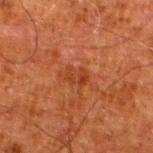Clinical impression: The lesion was photographed on a routine skin check and not biopsied; there is no pathology result. Background: Longest diameter approximately 2.5 mm. A male subject, aged around 80. A 15 mm close-up tile from a total-body photography series done for melanoma screening. Located on the left lower leg. Automated image analysis of the tile measured a footprint of about 3.5 mm², an outline eccentricity of about 0.75 (0 = round, 1 = elongated), and two-axis asymmetry of about 0.35. It also reported an average lesion color of about L≈33 a*≈25 b*≈33 (CIELAB) and about 6 CIELAB-L* units darker than the surrounding skin. The software also gave a classifier nevus-likeness of about 0/100 and a lesion-detection confidence of about 100/100. This is a cross-polarized tile.